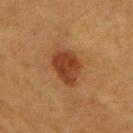<record>
  <automated_metrics>
    <cielab_L>33</cielab_L>
    <cielab_a>22</cielab_a>
    <cielab_b>30</cielab_b>
    <vs_skin_darker_L>10.0</vs_skin_darker_L>
    <color_variation_0_10>4.0</color_variation_0_10>
  </automated_metrics>
  <site>arm</site>
  <image>
    <source>total-body photography crop</source>
    <field_of_view_mm>15</field_of_view_mm>
  </image>
  <lesion_size>
    <long_diameter_mm_approx>4.0</long_diameter_mm_approx>
  </lesion_size>
  <lighting>cross-polarized</lighting>
  <patient>
    <sex>female</sex>
    <age_approx>55</age_approx>
  </patient>
</record>